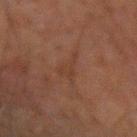notes: imaged on a skin check; not biopsied
image: total-body-photography crop, ~15 mm field of view
lighting: cross-polarized illumination
body site: the arm
patient: male, in their 60s
automated metrics: border irregularity of about 7 on a 0–10 scale, a within-lesion color-variation index near 0.5/10, and a peripheral color-asymmetry measure near 0; lesion-presence confidence of about 70/100
lesion diameter: about 4 mm